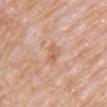{"biopsy_status": "not biopsied; imaged during a skin examination", "lighting": "white-light", "image": {"source": "total-body photography crop", "field_of_view_mm": 15}, "site": "front of the torso", "lesion_size": {"long_diameter_mm_approx": 3.0}, "patient": {"sex": "female", "age_approx": 60}}